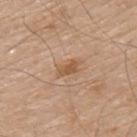* workup · total-body-photography surveillance lesion; no biopsy
* location · the mid back
* TBP lesion metrics · a mean CIELAB color near L≈55 a*≈20 b*≈34 and a normalized lesion–skin contrast near 7
* diameter · about 3 mm
* patient · male, aged 78 to 82
* image source · total-body-photography crop, ~15 mm field of view
* tile lighting · white-light illumination